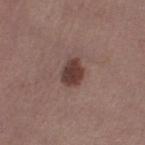Impression: Captured during whole-body skin photography for melanoma surveillance; the lesion was not biopsied. Clinical summary: The lesion's longest dimension is about 3 mm. A female subject approximately 50 years of age. A 15 mm close-up extracted from a 3D total-body photography capture. Automated image analysis of the tile measured an area of roughly 5.5 mm² and a shape-asymmetry score of about 0.2 (0 = symmetric). And it measured a border-irregularity rating of about 1.5/10, a color-variation rating of about 3/10, and radial color variation of about 1. It also reported an automated nevus-likeness rating near 95 out of 100 and lesion-presence confidence of about 100/100. Captured under white-light illumination. On the leg.